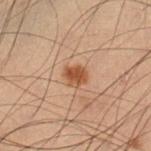The patient is a male about 55 years old. Longest diameter approximately 2.5 mm. Automated tile analysis of the lesion measured a lesion area of about 4.5 mm² and an outline eccentricity of about 0.5 (0 = round, 1 = elongated). And it measured an automated nevus-likeness rating near 95 out of 100 and a lesion-detection confidence of about 100/100. The lesion is located on the left lower leg. This is a cross-polarized tile. A roughly 15 mm field-of-view crop from a total-body skin photograph.